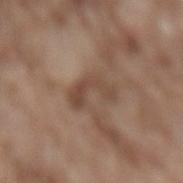This lesion was catalogued during total-body skin photography and was not selected for biopsy.
About 4.5 mm across.
A lesion tile, about 15 mm wide, cut from a 3D total-body photograph.
Captured under white-light illumination.
Automated tile analysis of the lesion measured a shape eccentricity near 0.85. It also reported roughly 9 lightness units darker than nearby skin and a lesion-to-skin contrast of about 7 (normalized; higher = more distinct).
On the lower back.
A male patient in their mid- to late 70s.